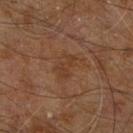This lesion was catalogued during total-body skin photography and was not selected for biopsy. The lesion is on the right leg. The total-body-photography lesion software estimated an average lesion color of about L≈36 a*≈19 b*≈29 (CIELAB) and a lesion–skin lightness drop of about 5. The analysis additionally found a nevus-likeness score of about 0/100 and a detector confidence of about 100 out of 100 that the crop contains a lesion. Imaged with cross-polarized lighting. A region of skin cropped from a whole-body photographic capture, roughly 15 mm wide. Measured at roughly 3 mm in maximum diameter. A male subject about 60 years old.